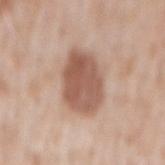Imaged during a routine full-body skin examination; the lesion was not biopsied and no histopathology is available. This is a white-light tile. Cropped from a whole-body photographic skin survey; the tile spans about 15 mm. The lesion is on the mid back. The lesion's longest dimension is about 6.5 mm. Automated tile analysis of the lesion measured an area of roughly 20 mm², an outline eccentricity of about 0.8 (0 = round, 1 = elongated), and two-axis asymmetry of about 0.15. And it measured a mean CIELAB color near L≈56 a*≈20 b*≈28. It also reported border irregularity of about 1.5 on a 0–10 scale, a within-lesion color-variation index near 4/10, and radial color variation of about 1.5. The software also gave lesion-presence confidence of about 100/100. A male patient about 50 years old.